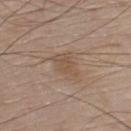Recorded during total-body skin imaging; not selected for excision or biopsy. The patient is a male in their 80s. On the chest. A 15 mm crop from a total-body photograph taken for skin-cancer surveillance.